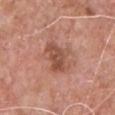Q: Was this lesion biopsied?
A: catalogued during a skin exam; not biopsied
Q: How large is the lesion?
A: ≈4.5 mm
Q: Patient demographics?
A: male, in their 60s
Q: What did automated image analysis measure?
A: a lesion area of about 10 mm² and an eccentricity of roughly 0.8; an average lesion color of about L≈51 a*≈24 b*≈29 (CIELAB), about 10 CIELAB-L* units darker than the surrounding skin, and a lesion-to-skin contrast of about 7 (normalized; higher = more distinct); a border-irregularity rating of about 4/10, a color-variation rating of about 4/10, and radial color variation of about 1.5; a nevus-likeness score of about 0/100 and a detector confidence of about 100 out of 100 that the crop contains a lesion
Q: What is the imaging modality?
A: ~15 mm tile from a whole-body skin photo
Q: Lesion location?
A: the chest
Q: How was the tile lit?
A: white-light